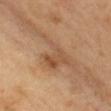Part of a total-body skin-imaging series; this lesion was reviewed on a skin check and was not flagged for biopsy. The lesion is located on the chest. The total-body-photography lesion software estimated a lesion area of about 12 mm², a shape eccentricity near 0.85, and a symmetry-axis asymmetry near 0.7. And it measured a lesion–skin lightness drop of about 8 and a lesion-to-skin contrast of about 6 (normalized; higher = more distinct). The analysis additionally found internal color variation of about 4.5 on a 0–10 scale and a peripheral color-asymmetry measure near 1. The software also gave a classifier nevus-likeness of about 0/100 and a detector confidence of about 95 out of 100 that the crop contains a lesion. A female subject aged around 40. Approximately 7.5 mm at its widest. Imaged with cross-polarized lighting. A region of skin cropped from a whole-body photographic capture, roughly 15 mm wide.